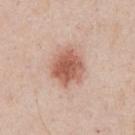Imaged with white-light lighting. A 15 mm close-up tile from a total-body photography series done for melanoma screening. An algorithmic analysis of the crop reported a lesion color around L≈59 a*≈23 b*≈29 in CIELAB, about 13 CIELAB-L* units darker than the surrounding skin, and a normalized border contrast of about 9. The software also gave internal color variation of about 4 on a 0–10 scale and a peripheral color-asymmetry measure near 1. From the front of the torso. A male patient approximately 50 years of age.